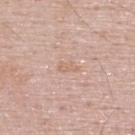This lesion was catalogued during total-body skin photography and was not selected for biopsy.
From the upper back.
Automated image analysis of the tile measured an average lesion color of about L≈65 a*≈18 b*≈29 (CIELAB), roughly 6 lightness units darker than nearby skin, and a lesion-to-skin contrast of about 4.5 (normalized; higher = more distinct). It also reported a nevus-likeness score of about 0/100 and a lesion-detection confidence of about 100/100.
A male subject aged 48–52.
This image is a 15 mm lesion crop taken from a total-body photograph.